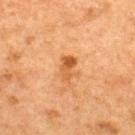Impression: No biopsy was performed on this lesion — it was imaged during a full skin examination and was not determined to be concerning. Image and clinical context: The lesion-visualizer software estimated a footprint of about 4.5 mm² and a symmetry-axis asymmetry near 0.3. And it measured a mean CIELAB color near L≈47 a*≈23 b*≈37, a lesion–skin lightness drop of about 9, and a normalized lesion–skin contrast near 7.5. The analysis additionally found border irregularity of about 3.5 on a 0–10 scale, internal color variation of about 4.5 on a 0–10 scale, and radial color variation of about 1.5. The software also gave a lesion-detection confidence of about 100/100. The subject is a male approximately 50 years of age. The lesion's longest dimension is about 3 mm. Located on the back. A roughly 15 mm field-of-view crop from a total-body skin photograph.